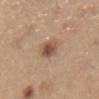The lesion was photographed on a routine skin check and not biopsied; there is no pathology result. A lesion tile, about 15 mm wide, cut from a 3D total-body photograph. The lesion is located on the abdomen. The subject is a male in their 70s. This is a white-light tile.